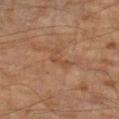Recorded during total-body skin imaging; not selected for excision or biopsy.
An algorithmic analysis of the crop reported a lesion color around L≈47 a*≈21 b*≈33 in CIELAB, about 6 CIELAB-L* units darker than the surrounding skin, and a normalized border contrast of about 5. And it measured a detector confidence of about 100 out of 100 that the crop contains a lesion.
A region of skin cropped from a whole-body photographic capture, roughly 15 mm wide.
The lesion is located on the left forearm.
A male patient aged 68–72.
The lesion's longest dimension is about 3 mm.
Captured under cross-polarized illumination.